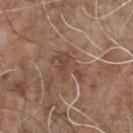Q: Was a biopsy performed?
A: catalogued during a skin exam; not biopsied
Q: Who is the patient?
A: male, in their 70s
Q: What kind of image is this?
A: total-body-photography crop, ~15 mm field of view
Q: What did automated image analysis measure?
A: a lesion-to-skin contrast of about 6 (normalized; higher = more distinct); a within-lesion color-variation index near 2/10 and a peripheral color-asymmetry measure near 1; a nevus-likeness score of about 0/100 and lesion-presence confidence of about 60/100
Q: Where on the body is the lesion?
A: the chest
Q: How large is the lesion?
A: ~4 mm (longest diameter)